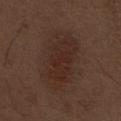{
  "biopsy_status": "not biopsied; imaged during a skin examination",
  "image": {
    "source": "total-body photography crop",
    "field_of_view_mm": 15
  },
  "lighting": "white-light",
  "site": "abdomen",
  "lesion_size": {
    "long_diameter_mm_approx": 8.0
  },
  "automated_metrics": {
    "area_mm2_approx": 26.0,
    "eccentricity": 0.85,
    "shape_asymmetry": 0.15,
    "cielab_L": 27,
    "cielab_a": 17,
    "cielab_b": 22,
    "vs_skin_darker_L": 6.0,
    "border_irregularity_0_10": 3.0,
    "peripheral_color_asymmetry": 1.0,
    "nevus_likeness_0_100": 75,
    "lesion_detection_confidence_0_100": 100
  },
  "patient": {
    "sex": "male",
    "age_approx": 70
  }
}